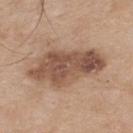Cropped from a whole-body photographic skin survey; the tile spans about 15 mm. On the mid back. This is a white-light tile. A male patient, aged approximately 75.